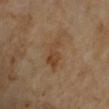<record>
  <biopsy_status>not biopsied; imaged during a skin examination</biopsy_status>
  <image>
    <source>total-body photography crop</source>
    <field_of_view_mm>15</field_of_view_mm>
  </image>
  <patient>
    <sex>male</sex>
    <age_approx>70</age_approx>
  </patient>
  <site>right upper arm</site>
  <lighting>cross-polarized</lighting>
</record>